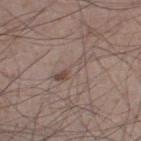Q: Was a biopsy performed?
A: catalogued during a skin exam; not biopsied
Q: What did automated image analysis measure?
A: an area of roughly 5.5 mm², a shape eccentricity near 0.95, and a shape-asymmetry score of about 0.3 (0 = symmetric); a mean CIELAB color near L≈49 a*≈14 b*≈21 and a normalized border contrast of about 5; border irregularity of about 4 on a 0–10 scale, internal color variation of about 6.5 on a 0–10 scale, and peripheral color asymmetry of about 1.5; a classifier nevus-likeness of about 0/100 and a lesion-detection confidence of about 80/100
Q: How was the tile lit?
A: white-light
Q: What kind of image is this?
A: 15 mm crop, total-body photography
Q: What is the lesion's diameter?
A: ~4 mm (longest diameter)
Q: Patient demographics?
A: male, aged 53 to 57
Q: Lesion location?
A: the left thigh